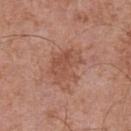notes=total-body-photography surveillance lesion; no biopsy
anatomic site=the front of the torso
patient=male, aged 68 to 72
illumination=white-light illumination
lesion size=~4.5 mm (longest diameter)
acquisition=~15 mm tile from a whole-body skin photo